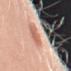<tbp_lesion>
<biopsy_status>not biopsied; imaged during a skin examination</biopsy_status>
<automated_metrics>
  <eccentricity>0.65</eccentricity>
  <shape_asymmetry>0.15</shape_asymmetry>
  <cielab_L>64</cielab_L>
  <cielab_a>25</cielab_a>
  <cielab_b>31</cielab_b>
  <vs_skin_darker_L>11.0</vs_skin_darker_L>
  <vs_skin_contrast_norm>6.5</vs_skin_contrast_norm>
  <color_variation_0_10>2.0</color_variation_0_10>
  <peripheral_color_asymmetry>0.5</peripheral_color_asymmetry>
  <nevus_likeness_0_100>95</nevus_likeness_0_100>
  <lesion_detection_confidence_0_100>100</lesion_detection_confidence_0_100>
</automated_metrics>
<lighting>white-light</lighting>
<lesion_size>
  <long_diameter_mm_approx>3.5</long_diameter_mm_approx>
</lesion_size>
<site>left upper arm</site>
<patient>
  <sex>female</sex>
  <age_approx>50</age_approx>
</patient>
<image>
  <source>total-body photography crop</source>
  <field_of_view_mm>15</field_of_view_mm>
</image>
</tbp_lesion>